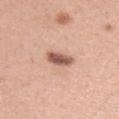Clinical impression: Part of a total-body skin-imaging series; this lesion was reviewed on a skin check and was not flagged for biopsy. Image and clinical context: The patient is a female aged approximately 30. The lesion is located on the left upper arm. A region of skin cropped from a whole-body photographic capture, roughly 15 mm wide.